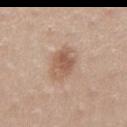Part of a total-body skin-imaging series; this lesion was reviewed on a skin check and was not flagged for biopsy.
A region of skin cropped from a whole-body photographic capture, roughly 15 mm wide.
The lesion is on the mid back.
Captured under white-light illumination.
About 4 mm across.
A male patient, approximately 35 years of age.